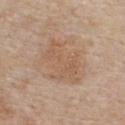Clinical impression: Captured during whole-body skin photography for melanoma surveillance; the lesion was not biopsied. Clinical summary: A male subject aged approximately 60. Imaged with white-light lighting. The lesion-visualizer software estimated a lesion color around L≈57 a*≈18 b*≈31 in CIELAB and a normalized lesion–skin contrast near 5. The software also gave border irregularity of about 8.5 on a 0–10 scale, a within-lesion color-variation index near 2.5/10, and radial color variation of about 1. The software also gave a classifier nevus-likeness of about 5/100 and a detector confidence of about 100 out of 100 that the crop contains a lesion. A region of skin cropped from a whole-body photographic capture, roughly 15 mm wide. About 6 mm across. On the chest.